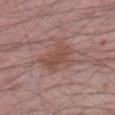Captured during whole-body skin photography for melanoma surveillance; the lesion was not biopsied. A male subject, approximately 55 years of age. On the leg. Captured under white-light illumination. The lesion-visualizer software estimated a border-irregularity rating of about 4.5/10 and a color-variation rating of about 2/10. Longest diameter approximately 4.5 mm. A region of skin cropped from a whole-body photographic capture, roughly 15 mm wide.